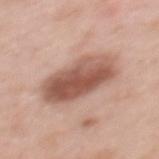Impression:
Part of a total-body skin-imaging series; this lesion was reviewed on a skin check and was not flagged for biopsy.
Image and clinical context:
A female patient aged 48–52. A lesion tile, about 15 mm wide, cut from a 3D total-body photograph. The lesion is on the mid back. An algorithmic analysis of the crop reported a lesion area of about 23 mm², an eccentricity of roughly 0.85, and a shape-asymmetry score of about 0.15 (0 = symmetric). The software also gave a within-lesion color-variation index near 6.5/10 and peripheral color asymmetry of about 2.5. About 7 mm across. Captured under white-light illumination.